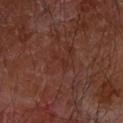Notes:
* follow-up: total-body-photography surveillance lesion; no biopsy
* size: about 2.5 mm
* imaging modality: ~15 mm tile from a whole-body skin photo
* automated metrics: a lesion color around L≈28 a*≈23 b*≈24 in CIELAB, about 4 CIELAB-L* units darker than the surrounding skin, and a normalized border contrast of about 4.5
* lighting: cross-polarized
* site: the left forearm
* patient: male, about 65 years old